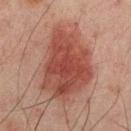Imaged during a routine full-body skin examination; the lesion was not biopsied and no histopathology is available.
The tile uses cross-polarized illumination.
A region of skin cropped from a whole-body photographic capture, roughly 15 mm wide.
The subject is a male aged 63–67.
The lesion is on the back.